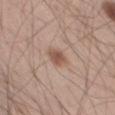biopsy status: total-body-photography surveillance lesion; no biopsy | illumination: white-light | image: 15 mm crop, total-body photography | subject: male, aged approximately 60 | diameter: ≈2.5 mm | location: the left thigh.